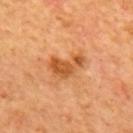Notes:
* biopsy status: imaged on a skin check; not biopsied
* automated metrics: a lesion area of about 8 mm² and an eccentricity of roughly 0.85; a mean CIELAB color near L≈55 a*≈28 b*≈45 and a normalized lesion–skin contrast near 8.5; a border-irregularity index near 6/10; an automated nevus-likeness rating near 25 out of 100 and lesion-presence confidence of about 100/100
* lighting: cross-polarized
* image: 15 mm crop, total-body photography
* lesion size: ≈4.5 mm
* site: the upper back
* subject: male, aged 63–67